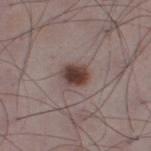Notes:
• TBP lesion metrics: an automated nevus-likeness rating near 100 out of 100
• tile lighting: white-light
• acquisition: 15 mm crop, total-body photography
• patient: male, about 50 years old
• site: the left lower leg
• diameter: ≈3 mm